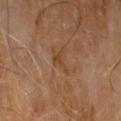workup = catalogued during a skin exam; not biopsied.